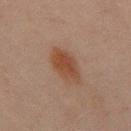{
  "biopsy_status": "not biopsied; imaged during a skin examination",
  "lighting": "cross-polarized",
  "patient": {
    "sex": "male",
    "age_approx": 45
  },
  "site": "mid back",
  "image": {
    "source": "total-body photography crop",
    "field_of_view_mm": 15
  },
  "automated_metrics": {
    "area_mm2_approx": 10.0,
    "eccentricity": 0.75,
    "shape_asymmetry": 0.2,
    "nevus_likeness_0_100": 100,
    "lesion_detection_confidence_0_100": 100
  },
  "lesion_size": {
    "long_diameter_mm_approx": 4.5
  }
}